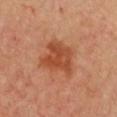notes: no biopsy performed (imaged during a skin exam)
acquisition: total-body-photography crop, ~15 mm field of view
patient: female, aged 38 to 42
tile lighting: cross-polarized
location: the front of the torso
size: ~4.5 mm (longest diameter)
automated lesion analysis: a footprint of about 14 mm², an outline eccentricity of about 0.5 (0 = round, 1 = elongated), and a shape-asymmetry score of about 0.35 (0 = symmetric)